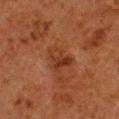Q: Where on the body is the lesion?
A: the leg
Q: Illumination type?
A: cross-polarized illumination
Q: How large is the lesion?
A: ≈3.5 mm
Q: What is the imaging modality?
A: total-body-photography crop, ~15 mm field of view
Q: Who is the patient?
A: male, roughly 80 years of age
Q: Automated lesion metrics?
A: a mean CIELAB color near L≈28 a*≈22 b*≈28 and a normalized lesion–skin contrast near 6.5; a border-irregularity rating of about 3.5/10 and peripheral color asymmetry of about 2.5; a classifier nevus-likeness of about 0/100 and a detector confidence of about 100 out of 100 that the crop contains a lesion